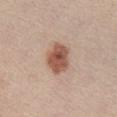The lesion was photographed on a routine skin check and not biopsied; there is no pathology result.
A 15 mm close-up tile from a total-body photography series done for melanoma screening.
Automated tile analysis of the lesion measured a border-irregularity index near 1.5/10 and a color-variation rating of about 4.5/10. The software also gave a classifier nevus-likeness of about 100/100 and a lesion-detection confidence of about 100/100.
Captured under white-light illumination.
The subject is a female aged approximately 35.
The lesion is on the front of the torso.
The lesion's longest dimension is about 4 mm.